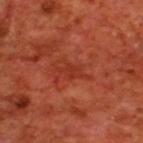<lesion>
  <biopsy_status>not biopsied; imaged during a skin examination</biopsy_status>
  <automated_metrics>
    <area_mm2_approx>3.0</area_mm2_approx>
    <eccentricity>0.8</eccentricity>
    <shape_asymmetry>0.4</shape_asymmetry>
    <cielab_L>35</cielab_L>
    <cielab_a>34</cielab_a>
    <cielab_b>35</cielab_b>
    <vs_skin_darker_L>6.0</vs_skin_darker_L>
    <vs_skin_contrast_norm>5.0</vs_skin_contrast_norm>
    <border_irregularity_0_10>4.0</border_irregularity_0_10>
    <color_variation_0_10>1.0</color_variation_0_10>
    <peripheral_color_asymmetry>0.5</peripheral_color_asymmetry>
    <nevus_likeness_0_100>0</nevus_likeness_0_100>
    <lesion_detection_confidence_0_100>80</lesion_detection_confidence_0_100>
  </automated_metrics>
  <patient>
    <sex>male</sex>
    <age_approx>70</age_approx>
  </patient>
  <site>upper back</site>
  <lesion_size>
    <long_diameter_mm_approx>2.5</long_diameter_mm_approx>
  </lesion_size>
  <image>
    <source>total-body photography crop</source>
    <field_of_view_mm>15</field_of_view_mm>
  </image>
  <lighting>cross-polarized</lighting>
</lesion>